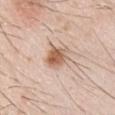patient:
  sex: male
  age_approx: 30
lighting: white-light
image:
  source: total-body photography crop
  field_of_view_mm: 15
automated_metrics:
  vs_skin_contrast_norm: 9.0
  border_irregularity_0_10: 2.5
  color_variation_0_10: 6.0
  peripheral_color_asymmetry: 2.0
  nevus_likeness_0_100: 95
  lesion_detection_confidence_0_100: 100
site: left upper arm
lesion_size:
  long_diameter_mm_approx: 3.5